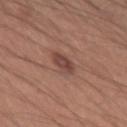biopsy_status: not biopsied; imaged during a skin examination
automated_metrics:
  eccentricity: 0.55
  shape_asymmetry: 0.2
  cielab_L: 44
  cielab_a: 21
  cielab_b: 24
  vs_skin_darker_L: 9.0
  vs_skin_contrast_norm: 7.5
  lesion_detection_confidence_0_100: 100
site: left upper arm
patient:
  sex: male
  age_approx: 40
lesion_size:
  long_diameter_mm_approx: 3.0
image:
  source: total-body photography crop
  field_of_view_mm: 15